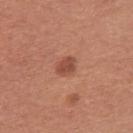Impression:
Captured during whole-body skin photography for melanoma surveillance; the lesion was not biopsied.
Context:
Captured under white-light illumination. A female patient, aged 48 to 52. On the upper back. Longest diameter approximately 2.5 mm. A 15 mm crop from a total-body photograph taken for skin-cancer surveillance.